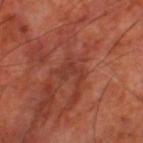Imaged during a routine full-body skin examination; the lesion was not biopsied and no histopathology is available. The lesion-visualizer software estimated an eccentricity of roughly 0.7 and a symmetry-axis asymmetry near 0.6. It also reported a lesion–skin lightness drop of about 6 and a normalized border contrast of about 5. From the left thigh. This image is a 15 mm lesion crop taken from a total-body photograph. A subject aged 63 to 67. Captured under cross-polarized illumination.